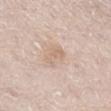follow-up: total-body-photography surveillance lesion; no biopsy
TBP lesion metrics: a footprint of about 4.5 mm², an outline eccentricity of about 0.9 (0 = round, 1 = elongated), and a shape-asymmetry score of about 0.4 (0 = symmetric)
anatomic site: the left thigh
subject: male, roughly 80 years of age
size: ≈3.5 mm
image: ~15 mm crop, total-body skin-cancer survey